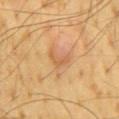| field | value |
|---|---|
| workup | imaged on a skin check; not biopsied |
| location | the mid back |
| subject | male, roughly 65 years of age |
| image | ~15 mm crop, total-body skin-cancer survey |
| lesion size | ≈3 mm |
| automated lesion analysis | a lesion area of about 3 mm² and an eccentricity of roughly 0.85; a border-irregularity rating of about 6.5/10, a within-lesion color-variation index near 0/10, and radial color variation of about 0; an automated nevus-likeness rating near 0 out of 100 and a detector confidence of about 100 out of 100 that the crop contains a lesion |